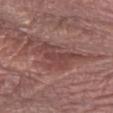Impression: Recorded during total-body skin imaging; not selected for excision or biopsy. Image and clinical context: On the right forearm. Approximately 5 mm at its widest. The patient is a female aged 63–67. This image is a 15 mm lesion crop taken from a total-body photograph.